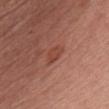Clinical impression: No biopsy was performed on this lesion — it was imaged during a full skin examination and was not determined to be concerning. Background: A region of skin cropped from a whole-body photographic capture, roughly 15 mm wide. The tile uses white-light illumination. The lesion-visualizer software estimated a classifier nevus-likeness of about 25/100 and a detector confidence of about 100 out of 100 that the crop contains a lesion. The subject is a male aged approximately 60. Longest diameter approximately 2.5 mm. Located on the chest.